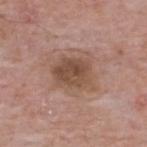notes: total-body-photography surveillance lesion; no biopsy
subject: male, in their mid- to late 60s
anatomic site: the upper back
diameter: ~5 mm (longest diameter)
acquisition: ~15 mm crop, total-body skin-cancer survey